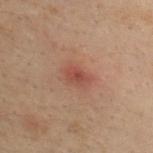workup: catalogued during a skin exam; not biopsied
image source: ~15 mm crop, total-body skin-cancer survey
site: the upper back
subject: male, aged 28–32